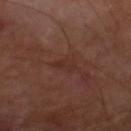notes = no biopsy performed (imaged during a skin exam)
site = the right lower leg
subject = male, about 70 years old
image source = total-body-photography crop, ~15 mm field of view
automated metrics = a border-irregularity index near 3.5/10, a within-lesion color-variation index near 0/10, and a peripheral color-asymmetry measure near 0; a classifier nevus-likeness of about 0/100